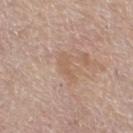Imaged during a routine full-body skin examination; the lesion was not biopsied and no histopathology is available.
A female subject about 65 years old.
Located on the right thigh.
The lesion's longest dimension is about 3.5 mm.
A 15 mm close-up tile from a total-body photography series done for melanoma screening.
The total-body-photography lesion software estimated an area of roughly 4 mm², an outline eccentricity of about 0.95 (0 = round, 1 = elongated), and two-axis asymmetry of about 0.35. The software also gave a lesion color around L≈58 a*≈17 b*≈29 in CIELAB and a normalized border contrast of about 4.5.